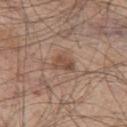follow-up: catalogued during a skin exam; not biopsied
image source: ~15 mm tile from a whole-body skin photo
location: the left thigh
subject: male, aged around 45
lighting: white-light illumination
size: ≈2.5 mm